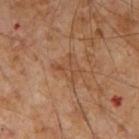The lesion was tiled from a total-body skin photograph and was not biopsied. An algorithmic analysis of the crop reported an area of roughly 5 mm², an outline eccentricity of about 0.55 (0 = round, 1 = elongated), and a symmetry-axis asymmetry near 0.45. The software also gave an automated nevus-likeness rating near 0 out of 100. A 15 mm close-up tile from a total-body photography series done for melanoma screening. A male patient aged approximately 55. Longest diameter approximately 3 mm. This is a cross-polarized tile. The lesion is on the arm.